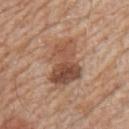Background:
From the right upper arm. A 15 mm close-up tile from a total-body photography series done for melanoma screening. A male patient about 60 years old. The lesion's longest dimension is about 6 mm. An algorithmic analysis of the crop reported a shape-asymmetry score of about 0.4 (0 = symmetric). It also reported a mean CIELAB color near L≈50 a*≈21 b*≈30, about 12 CIELAB-L* units darker than the surrounding skin, and a normalized border contrast of about 8.5. And it measured an automated nevus-likeness rating near 80 out of 100.